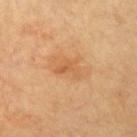Impression:
Part of a total-body skin-imaging series; this lesion was reviewed on a skin check and was not flagged for biopsy.
Clinical summary:
Automated image analysis of the tile measured a detector confidence of about 100 out of 100 that the crop contains a lesion. This is a cross-polarized tile. The patient is a female aged approximately 70. From the arm. A lesion tile, about 15 mm wide, cut from a 3D total-body photograph.